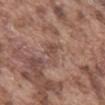{"biopsy_status": "not biopsied; imaged during a skin examination", "lighting": "white-light", "patient": {"sex": "male", "age_approx": 75}, "lesion_size": {"long_diameter_mm_approx": 3.5}, "automated_metrics": {"border_irregularity_0_10": 5.5, "color_variation_0_10": 2.0, "peripheral_color_asymmetry": 0.5, "nevus_likeness_0_100": 0}, "site": "abdomen", "image": {"source": "total-body photography crop", "field_of_view_mm": 15}}